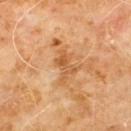Recorded during total-body skin imaging; not selected for excision or biopsy.
A male subject, approximately 60 years of age.
The lesion's longest dimension is about 4 mm.
Imaged with cross-polarized lighting.
A close-up tile cropped from a whole-body skin photograph, about 15 mm across.
The lesion is located on the chest.
Automated tile analysis of the lesion measured a footprint of about 6.5 mm² and a shape eccentricity near 0.7. The software also gave an average lesion color of about L≈55 a*≈23 b*≈41 (CIELAB), about 8 CIELAB-L* units darker than the surrounding skin, and a normalized lesion–skin contrast near 6. The software also gave a within-lesion color-variation index near 5.5/10.